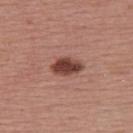Q: Was a biopsy performed?
A: total-body-photography surveillance lesion; no biopsy
Q: What is the imaging modality?
A: ~15 mm tile from a whole-body skin photo
Q: What lighting was used for the tile?
A: white-light
Q: Lesion size?
A: ≈4 mm
Q: Patient demographics?
A: female, approximately 55 years of age
Q: What did automated image analysis measure?
A: a footprint of about 7 mm², an eccentricity of roughly 0.8, and a shape-asymmetry score of about 0.15 (0 = symmetric); a border-irregularity index near 1.5/10, internal color variation of about 3.5 on a 0–10 scale, and a peripheral color-asymmetry measure near 1; a classifier nevus-likeness of about 100/100 and a lesion-detection confidence of about 100/100
Q: Lesion location?
A: the upper back